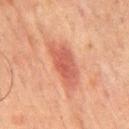Clinical impression:
This lesion was catalogued during total-body skin photography and was not selected for biopsy.
Acquisition and patient details:
The patient is a male aged 63–67. The tile uses cross-polarized illumination. A lesion tile, about 15 mm wide, cut from a 3D total-body photograph. Located on the back. Approximately 6 mm at its widest.